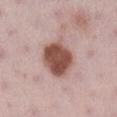The lesion was photographed on a routine skin check and not biopsied; there is no pathology result. A female patient approximately 30 years of age. Approximately 5 mm at its widest. An algorithmic analysis of the crop reported a footprint of about 16 mm², an outline eccentricity of about 0.55 (0 = round, 1 = elongated), and two-axis asymmetry of about 0.15. The analysis additionally found a border-irregularity index near 1.5/10 and internal color variation of about 4.5 on a 0–10 scale. This is a white-light tile. From the right lower leg. Cropped from a total-body skin-imaging series; the visible field is about 15 mm.